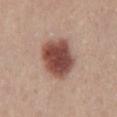Assessment:
Captured during whole-body skin photography for melanoma surveillance; the lesion was not biopsied.
Acquisition and patient details:
The tile uses white-light illumination. The lesion is on the lower back. The patient is a male about 40 years old. A close-up tile cropped from a whole-body skin photograph, about 15 mm across. The lesion-visualizer software estimated a border-irregularity rating of about 1.5/10, a within-lesion color-variation index near 5.5/10, and a peripheral color-asymmetry measure near 1.5. Measured at roughly 5.5 mm in maximum diameter.